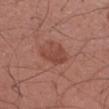No biopsy was performed on this lesion — it was imaged during a full skin examination and was not determined to be concerning.
A 15 mm close-up tile from a total-body photography series done for melanoma screening.
The lesion is located on the left forearm.
The tile uses white-light illumination.
A male patient in their mid- to late 30s.
The total-body-photography lesion software estimated a lesion-detection confidence of about 100/100.
Measured at roughly 4 mm in maximum diameter.